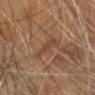Impression:
Part of a total-body skin-imaging series; this lesion was reviewed on a skin check and was not flagged for biopsy.
Clinical summary:
The tile uses cross-polarized illumination. A male subject aged approximately 75. The lesion's longest dimension is about 4 mm. On the head or neck. The total-body-photography lesion software estimated a border-irregularity rating of about 5.5/10 and a peripheral color-asymmetry measure near 1. A 15 mm crop from a total-body photograph taken for skin-cancer surveillance.